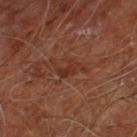Image and clinical context:
A male patient, in their 60s. The lesion is located on the leg. The tile uses cross-polarized illumination. The lesion-visualizer software estimated a lesion color around L≈31 a*≈22 b*≈28 in CIELAB, a lesion–skin lightness drop of about 6, and a lesion-to-skin contrast of about 6 (normalized; higher = more distinct). The software also gave a nevus-likeness score of about 0/100 and lesion-presence confidence of about 100/100. Cropped from a total-body skin-imaging series; the visible field is about 15 mm. Approximately 4 mm at its widest.